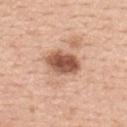Background: Cropped from a whole-body photographic skin survey; the tile spans about 15 mm. Imaged with white-light lighting. A male subject in their 50s. Measured at roughly 4.5 mm in maximum diameter. Located on the mid back.